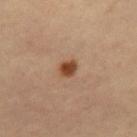Findings:
- anatomic site — the left leg
- imaging modality — total-body-photography crop, ~15 mm field of view
- patient — female, aged around 60